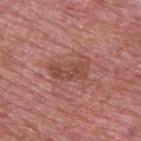Findings:
- follow-up: imaged on a skin check; not biopsied
- body site: the upper back
- TBP lesion metrics: a lesion area of about 8.5 mm², an outline eccentricity of about 0.85 (0 = round, 1 = elongated), and a symmetry-axis asymmetry near 0.3; internal color variation of about 2.5 on a 0–10 scale and peripheral color asymmetry of about 1
- patient: male, aged 73 to 77
- imaging modality: total-body-photography crop, ~15 mm field of view
- lesion size: ~4.5 mm (longest diameter)